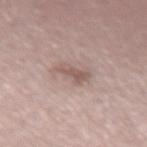| field | value |
|---|---|
| lighting | white-light |
| acquisition | total-body-photography crop, ~15 mm field of view |
| site | the left forearm |
| subject | female, aged approximately 45 |